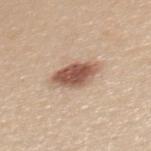Background:
Cropped from a total-body skin-imaging series; the visible field is about 15 mm. The patient is a female aged 23 to 27. On the upper back. This is a white-light tile.